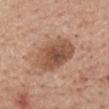Notes:
– workup: imaged on a skin check; not biopsied
– anatomic site: the mid back
– subject: male, roughly 55 years of age
– image source: ~15 mm tile from a whole-body skin photo
– tile lighting: white-light
– diameter: ≈6 mm